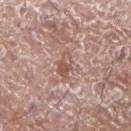Q: Was this lesion biopsied?
A: total-body-photography surveillance lesion; no biopsy
Q: How was this image acquired?
A: total-body-photography crop, ~15 mm field of view
Q: Illumination type?
A: white-light
Q: What is the anatomic site?
A: the arm
Q: How large is the lesion?
A: about 3.5 mm
Q: What did automated image analysis measure?
A: a mean CIELAB color near L≈54 a*≈21 b*≈26, about 10 CIELAB-L* units darker than the surrounding skin, and a normalized border contrast of about 7; a border-irregularity rating of about 3.5/10, internal color variation of about 3 on a 0–10 scale, and peripheral color asymmetry of about 1; an automated nevus-likeness rating near 0 out of 100 and lesion-presence confidence of about 95/100
Q: Patient demographics?
A: male, aged approximately 75